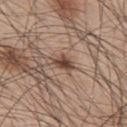<case>
  <biopsy_status>not biopsied; imaged during a skin examination</biopsy_status>
  <lighting>white-light</lighting>
  <automated_metrics>
    <area_mm2_approx>4.0</area_mm2_approx>
    <eccentricity>0.75</eccentricity>
    <shape_asymmetry>0.25</shape_asymmetry>
    <cielab_L>45</cielab_L>
    <cielab_a>18</cielab_a>
    <cielab_b>26</cielab_b>
    <vs_skin_darker_L>13.0</vs_skin_darker_L>
    <vs_skin_contrast_norm>10.0</vs_skin_contrast_norm>
    <border_irregularity_0_10>2.0</border_irregularity_0_10>
    <color_variation_0_10>2.5</color_variation_0_10>
    <nevus_likeness_0_100>90</nevus_likeness_0_100>
  </automated_metrics>
  <image>
    <source>total-body photography crop</source>
    <field_of_view_mm>15</field_of_view_mm>
  </image>
  <patient>
    <sex>male</sex>
    <age_approx>45</age_approx>
  </patient>
  <site>upper back</site>
  <lesion_size>
    <long_diameter_mm_approx>2.5</long_diameter_mm_approx>
  </lesion_size>
</case>